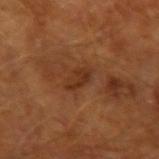biopsy_status: not biopsied; imaged during a skin examination
site: left forearm
patient:
  sex: male
  age_approx: 65
image:
  source: total-body photography crop
  field_of_view_mm: 15
lighting: cross-polarized